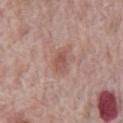workup: total-body-photography surveillance lesion; no biopsy | acquisition: ~15 mm crop, total-body skin-cancer survey | TBP lesion metrics: a lesion area of about 4 mm², an outline eccentricity of about 0.75 (0 = round, 1 = elongated), and a shape-asymmetry score of about 0.25 (0 = symmetric); a mean CIELAB color near L≈53 a*≈21 b*≈25, a lesion–skin lightness drop of about 8, and a lesion-to-skin contrast of about 6 (normalized; higher = more distinct); a nevus-likeness score of about 55/100 | patient: male, about 70 years old | lesion size: about 2.5 mm | location: the abdomen.